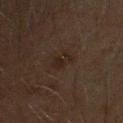Q: Was this lesion biopsied?
A: imaged on a skin check; not biopsied
Q: How large is the lesion?
A: ≈2.5 mm
Q: How was this image acquired?
A: ~15 mm tile from a whole-body skin photo
Q: Patient demographics?
A: male, roughly 70 years of age
Q: Lesion location?
A: the head or neck
Q: How was the tile lit?
A: cross-polarized illumination